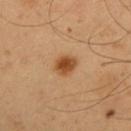The lesion was tiled from a total-body skin photograph and was not biopsied. Imaged with cross-polarized lighting. About 3 mm across. This image is a 15 mm lesion crop taken from a total-body photograph. A male subject, in their mid- to late 50s. The lesion is located on the arm.